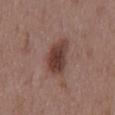Impression: The lesion was photographed on a routine skin check and not biopsied; there is no pathology result. Context: Longest diameter approximately 5 mm. The patient is a male aged 48–52. The lesion-visualizer software estimated roughly 12 lightness units darker than nearby skin and a normalized lesion–skin contrast near 10. The software also gave border irregularity of about 2.5 on a 0–10 scale and a peripheral color-asymmetry measure near 1.5. The tile uses white-light illumination. A 15 mm close-up tile from a total-body photography series done for melanoma screening. Located on the mid back.